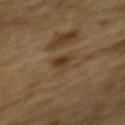Q: Illumination type?
A: cross-polarized
Q: How was this image acquired?
A: 15 mm crop, total-body photography
Q: Who is the patient?
A: male, about 85 years old or older
Q: Lesion location?
A: the back